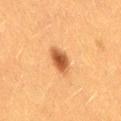notes: total-body-photography surveillance lesion; no biopsy
automated metrics: an area of roughly 6 mm² and an outline eccentricity of about 0.75 (0 = round, 1 = elongated); a border-irregularity rating of about 2/10 and internal color variation of about 3.5 on a 0–10 scale
tile lighting: cross-polarized illumination
subject: female, aged 38–42
image source: 15 mm crop, total-body photography
anatomic site: the leg
lesion diameter: about 3.5 mm